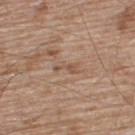{"biopsy_status": "not biopsied; imaged during a skin examination", "image": {"source": "total-body photography crop", "field_of_view_mm": 15}, "lighting": "white-light", "automated_metrics": {"area_mm2_approx": 3.5, "eccentricity": 0.9, "cielab_L": 53, "cielab_a": 17, "cielab_b": 28, "vs_skin_darker_L": 7.0, "vs_skin_contrast_norm": 5.0, "border_irregularity_0_10": 5.5, "color_variation_0_10": 1.0, "peripheral_color_asymmetry": 0.5, "nevus_likeness_0_100": 0, "lesion_detection_confidence_0_100": 80}, "site": "upper back", "patient": {"sex": "male", "age_approx": 65}, "lesion_size": {"long_diameter_mm_approx": 3.0}}